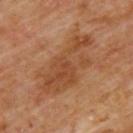biopsy_status: not biopsied; imaged during a skin examination
patient:
  sex: male
  age_approx: 60
lesion_size:
  long_diameter_mm_approx: 8.0
lighting: cross-polarized
site: upper back
image:
  source: total-body photography crop
  field_of_view_mm: 15